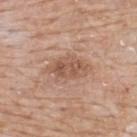Part of a total-body skin-imaging series; this lesion was reviewed on a skin check and was not flagged for biopsy. A 15 mm crop from a total-body photograph taken for skin-cancer surveillance. Imaged with white-light lighting. Approximately 5 mm at its widest. The total-body-photography lesion software estimated roughly 9 lightness units darker than nearby skin and a lesion-to-skin contrast of about 6.5 (normalized; higher = more distinct). The software also gave an automated nevus-likeness rating near 10 out of 100 and lesion-presence confidence of about 100/100. A male patient aged approximately 65. On the upper back.